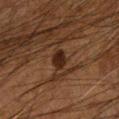No biopsy was performed on this lesion — it was imaged during a full skin examination and was not determined to be concerning.
The tile uses cross-polarized illumination.
A roughly 15 mm field-of-view crop from a total-body skin photograph.
Located on the left forearm.
A male subject aged 63–67.